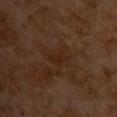Part of a total-body skin-imaging series; this lesion was reviewed on a skin check and was not flagged for biopsy. A 15 mm crop from a total-body photograph taken for skin-cancer surveillance. A male patient roughly 60 years of age. From the chest. This is a cross-polarized tile. Automated image analysis of the tile measured a lesion area of about 3.5 mm², an eccentricity of roughly 0.85, and a symmetry-axis asymmetry near 0.4.